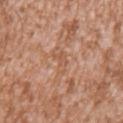{
  "biopsy_status": "not biopsied; imaged during a skin examination",
  "site": "left upper arm",
  "patient": {
    "sex": "male",
    "age_approx": 45
  },
  "image": {
    "source": "total-body photography crop",
    "field_of_view_mm": 15
  }
}